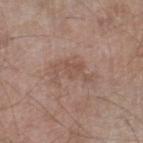| field | value |
|---|---|
| notes | imaged on a skin check; not biopsied |
| TBP lesion metrics | a footprint of about 11 mm², an outline eccentricity of about 0.85 (0 = round, 1 = elongated), and two-axis asymmetry of about 0.5; internal color variation of about 2.5 on a 0–10 scale; a nevus-likeness score of about 0/100 and lesion-presence confidence of about 100/100 |
| tile lighting | white-light illumination |
| size | ≈5 mm |
| acquisition | ~15 mm crop, total-body skin-cancer survey |
| subject | male, aged approximately 60 |
| location | the right lower leg |